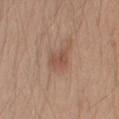  biopsy_status: not biopsied; imaged during a skin examination
  patient:
    sex: male
    age_approx: 20
  site: right upper arm
  lesion_size:
    long_diameter_mm_approx: 3.0
  lighting: white-light
  image:
    source: total-body photography crop
    field_of_view_mm: 15
  automated_metrics:
    area_mm2_approx: 4.5
    eccentricity: 0.65
    shape_asymmetry: 0.3